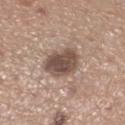The lesion was tiled from a total-body skin photograph and was not biopsied.
Measured at roughly 4 mm in maximum diameter.
The patient is a female aged around 55.
This image is a 15 mm lesion crop taken from a total-body photograph.
On the left lower leg.
The tile uses white-light illumination.
The total-body-photography lesion software estimated a classifier nevus-likeness of about 50/100.